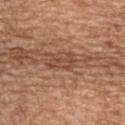No biopsy was performed on this lesion — it was imaged during a full skin examination and was not determined to be concerning. A 15 mm crop from a total-body photograph taken for skin-cancer surveillance. The lesion is located on the upper back. A female patient, roughly 55 years of age.